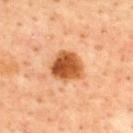biopsy status = imaged on a skin check; not biopsied
image = ~15 mm crop, total-body skin-cancer survey
automated metrics = a classifier nevus-likeness of about 95/100
diameter = ~4 mm (longest diameter)
tile lighting = cross-polarized illumination
location = the upper back
patient = male, aged approximately 65